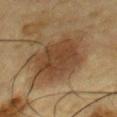Impression:
Part of a total-body skin-imaging series; this lesion was reviewed on a skin check and was not flagged for biopsy.
Acquisition and patient details:
A 15 mm crop from a total-body photograph taken for skin-cancer surveillance. The lesion is located on the front of the torso. The tile uses cross-polarized illumination. Measured at roughly 7.5 mm in maximum diameter. A male patient approximately 85 years of age or older.